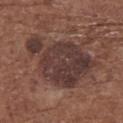notes=imaged on a skin check; not biopsied
subject=female, aged 73–77
location=the back
image source=15 mm crop, total-body photography